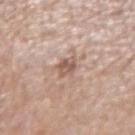Q: Was this lesion biopsied?
A: catalogued during a skin exam; not biopsied
Q: What is the lesion's diameter?
A: ~2.5 mm (longest diameter)
Q: How was this image acquired?
A: 15 mm crop, total-body photography
Q: Patient demographics?
A: female, in their 70s
Q: Automated lesion metrics?
A: an outline eccentricity of about 0.6 (0 = round, 1 = elongated) and a symmetry-axis asymmetry near 0.2; border irregularity of about 2 on a 0–10 scale, internal color variation of about 3 on a 0–10 scale, and radial color variation of about 1
Q: Where on the body is the lesion?
A: the left forearm
Q: Illumination type?
A: white-light illumination